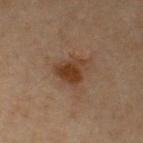On the chest.
A male patient aged 58–62.
A 15 mm close-up extracted from a 3D total-body photography capture.
The tile uses cross-polarized illumination.